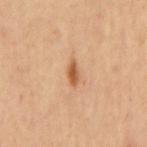follow-up: no biopsy performed (imaged during a skin exam) | imaging modality: 15 mm crop, total-body photography | body site: the mid back | patient: male, approximately 60 years of age.